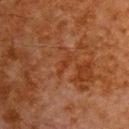The lesion was photographed on a routine skin check and not biopsied; there is no pathology result. A male subject aged 78 to 82. Automated image analysis of the tile measured an outline eccentricity of about 0.95 (0 = round, 1 = elongated) and a symmetry-axis asymmetry near 0.45. It also reported a border-irregularity rating of about 5/10, a color-variation rating of about 0/10, and radial color variation of about 0. The analysis additionally found a nevus-likeness score of about 0/100. Longest diameter approximately 2.5 mm. Located on the chest. Cropped from a whole-body photographic skin survey; the tile spans about 15 mm. Captured under cross-polarized illumination.